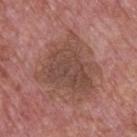biopsy_status: not biopsied; imaged during a skin examination
image:
  source: total-body photography crop
  field_of_view_mm: 15
site: upper back
patient:
  sex: male
  age_approx: 75
lighting: white-light